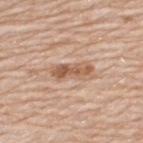Imaged with white-light lighting.
The subject is a male aged 78–82.
Located on the back.
A lesion tile, about 15 mm wide, cut from a 3D total-body photograph.
About 4.5 mm across.